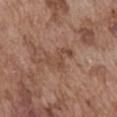Findings:
* notes — imaged on a skin check; not biopsied
* site — the abdomen
* size — about 4 mm
* patient — male, aged approximately 75
* lighting — white-light illumination
* acquisition — total-body-photography crop, ~15 mm field of view